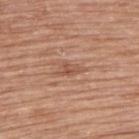The lesion was tiled from a total-body skin photograph and was not biopsied. From the upper back. A female subject in their 60s. The total-body-photography lesion software estimated a lesion color around L≈53 a*≈22 b*≈31 in CIELAB, roughly 8 lightness units darker than nearby skin, and a lesion-to-skin contrast of about 6 (normalized; higher = more distinct). And it measured a classifier nevus-likeness of about 0/100 and a lesion-detection confidence of about 95/100. Cropped from a total-body skin-imaging series; the visible field is about 15 mm. Captured under white-light illumination. Longest diameter approximately 2.5 mm.